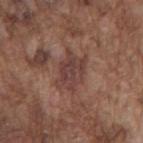Imaged during a routine full-body skin examination; the lesion was not biopsied and no histopathology is available. The lesion is on the back. A male subject aged 73–77. This is a white-light tile. This image is a 15 mm lesion crop taken from a total-body photograph.